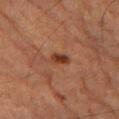  image:
    source: total-body photography crop
    field_of_view_mm: 15
  automated_metrics:
    area_mm2_approx: 3.5
    eccentricity: 0.75
    shape_asymmetry: 0.15
    border_irregularity_0_10: 1.5
    color_variation_0_10: 2.5
    peripheral_color_asymmetry: 1.0
    nevus_likeness_0_100: 90
    lesion_detection_confidence_0_100: 100
  patient:
    sex: male
    age_approx: 85+
  site: left thigh
  lesion_size:
    long_diameter_mm_approx: 2.5